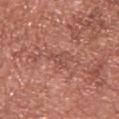Q: Was this lesion biopsied?
A: total-body-photography surveillance lesion; no biopsy
Q: What is the anatomic site?
A: the chest
Q: Automated lesion metrics?
A: a footprint of about 2.5 mm²; an average lesion color of about L≈50 a*≈25 b*≈27 (CIELAB), a lesion–skin lightness drop of about 6, and a normalized border contrast of about 4.5
Q: What is the imaging modality?
A: ~15 mm crop, total-body skin-cancer survey
Q: Who is the patient?
A: male, in their mid-40s
Q: What lighting was used for the tile?
A: white-light